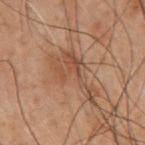Clinical impression:
No biopsy was performed on this lesion — it was imaged during a full skin examination and was not determined to be concerning.
Acquisition and patient details:
The lesion is on the chest. This is a cross-polarized tile. The subject is a male in their 70s. The total-body-photography lesion software estimated a lesion color around L≈37 a*≈16 b*≈24 in CIELAB and roughly 6 lightness units darker than nearby skin. And it measured a within-lesion color-variation index near 3.5/10 and radial color variation of about 1. The software also gave a nevus-likeness score of about 0/100 and a detector confidence of about 100 out of 100 that the crop contains a lesion. Longest diameter approximately 7 mm. Cropped from a total-body skin-imaging series; the visible field is about 15 mm.